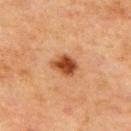<lesion>
  <biopsy_status>not biopsied; imaged during a skin examination</biopsy_status>
  <lesion_size>
    <long_diameter_mm_approx>3.0</long_diameter_mm_approx>
  </lesion_size>
  <site>back</site>
  <image>
    <source>total-body photography crop</source>
    <field_of_view_mm>15</field_of_view_mm>
  </image>
  <lighting>cross-polarized</lighting>
  <patient>
    <sex>male</sex>
    <age_approx>70</age_approx>
  </patient>
</lesion>